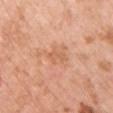Recorded during total-body skin imaging; not selected for excision or biopsy. A female subject, aged around 50. A lesion tile, about 15 mm wide, cut from a 3D total-body photograph. Automated tile analysis of the lesion measured an average lesion color of about L≈62 a*≈25 b*≈35 (CIELAB), a lesion–skin lightness drop of about 7, and a normalized lesion–skin contrast near 5. The analysis additionally found a border-irregularity index near 4/10 and a peripheral color-asymmetry measure near 0. It also reported an automated nevus-likeness rating near 0 out of 100 and a detector confidence of about 100 out of 100 that the crop contains a lesion. Captured under white-light illumination. The lesion is located on the chest. The lesion's longest dimension is about 2.5 mm.